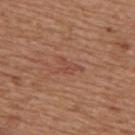The lesion was photographed on a routine skin check and not biopsied; there is no pathology result.
The patient is a male about 65 years old.
The lesion is located on the mid back.
Cropped from a total-body skin-imaging series; the visible field is about 15 mm.
The tile uses white-light illumination.
Measured at roughly 3 mm in maximum diameter.